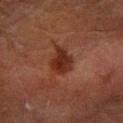The lesion was photographed on a routine skin check and not biopsied; there is no pathology result.
The patient is a male aged around 60.
A region of skin cropped from a whole-body photographic capture, roughly 15 mm wide.
Approximately 3.5 mm at its widest.
The lesion is located on the right forearm.
The tile uses cross-polarized illumination.